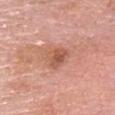Captured during whole-body skin photography for melanoma surveillance; the lesion was not biopsied. The recorded lesion diameter is about 3.5 mm. The lesion is on the head or neck. Cropped from a total-body skin-imaging series; the visible field is about 15 mm. This is a white-light tile. The subject is a male aged 78–82.